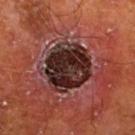| feature | finding |
|---|---|
| biopsy status | no biopsy performed (imaged during a skin exam) |
| TBP lesion metrics | a symmetry-axis asymmetry near 0.15; an average lesion color of about L≈25 a*≈19 b*≈19 (CIELAB) and a lesion-to-skin contrast of about 15 (normalized; higher = more distinct) |
| body site | the leg |
| lighting | cross-polarized illumination |
| imaging modality | ~15 mm crop, total-body skin-cancer survey |
| subject | male, roughly 65 years of age |